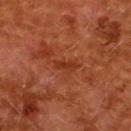Part of a total-body skin-imaging series; this lesion was reviewed on a skin check and was not flagged for biopsy.
Located on the upper back.
A roughly 15 mm field-of-view crop from a total-body skin photograph.
Approximately 2.5 mm at its widest.
A female subject, aged approximately 50.